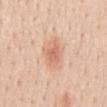Imaged during a routine full-body skin examination; the lesion was not biopsied and no histopathology is available.
The lesion's longest dimension is about 3.5 mm.
Captured under white-light illumination.
A lesion tile, about 15 mm wide, cut from a 3D total-body photograph.
The subject is a female roughly 45 years of age.
The lesion is on the mid back.